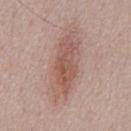workup: no biopsy performed (imaged during a skin exam) | patient: male, aged approximately 50 | anatomic site: the chest | image source: 15 mm crop, total-body photography | lesion size: ~8 mm (longest diameter).